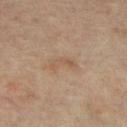Cropped from a whole-body photographic skin survey; the tile spans about 15 mm.
A female patient about 65 years old.
From the right lower leg.
The lesion's longest dimension is about 3 mm.